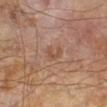This lesion was catalogued during total-body skin photography and was not selected for biopsy. The total-body-photography lesion software estimated a border-irregularity rating of about 4/10, a within-lesion color-variation index near 0.5/10, and a peripheral color-asymmetry measure near 0. The software also gave an automated nevus-likeness rating near 0 out of 100 and a detector confidence of about 100 out of 100 that the crop contains a lesion. A male subject aged approximately 65. A lesion tile, about 15 mm wide, cut from a 3D total-body photograph. This is a cross-polarized tile. About 2.5 mm across.